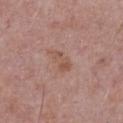biopsy status: total-body-photography surveillance lesion; no biopsy | lighting: white-light | acquisition: ~15 mm tile from a whole-body skin photo | site: the chest | automated metrics: an area of roughly 4 mm², an outline eccentricity of about 0.85 (0 = round, 1 = elongated), and two-axis asymmetry of about 0.3; about 7 CIELAB-L* units darker than the surrounding skin and a lesion-to-skin contrast of about 6 (normalized; higher = more distinct); a border-irregularity rating of about 3.5/10 and peripheral color asymmetry of about 0.5; a nevus-likeness score of about 0/100 and a lesion-detection confidence of about 100/100 | patient: male, roughly 65 years of age.